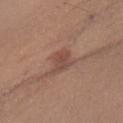{
  "biopsy_status": "not biopsied; imaged during a skin examination",
  "lighting": "white-light",
  "patient": {
    "sex": "female",
    "age_approx": 45
  },
  "site": "front of the torso",
  "automated_metrics": {
    "area_mm2_approx": 6.5,
    "eccentricity": 0.8,
    "shape_asymmetry": 0.3,
    "border_irregularity_0_10": 3.5,
    "color_variation_0_10": 2.0
  },
  "image": {
    "source": "total-body photography crop",
    "field_of_view_mm": 15
  }
}